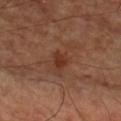No biopsy was performed on this lesion — it was imaged during a full skin examination and was not determined to be concerning. Cropped from a whole-body photographic skin survey; the tile spans about 15 mm. Located on the left forearm. The subject is aged 63–67.